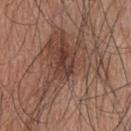Q: Is there a histopathology result?
A: total-body-photography surveillance lesion; no biopsy
Q: What kind of image is this?
A: ~15 mm crop, total-body skin-cancer survey
Q: Automated lesion metrics?
A: a lesion area of about 55 mm² and an eccentricity of roughly 0.85; a mean CIELAB color near L≈45 a*≈18 b*≈25, roughly 8 lightness units darker than nearby skin, and a lesion-to-skin contrast of about 6.5 (normalized; higher = more distinct); a border-irregularity rating of about 9/10 and radial color variation of about 2; a classifier nevus-likeness of about 65/100
Q: What lighting was used for the tile?
A: white-light
Q: Lesion size?
A: ~15 mm (longest diameter)
Q: Who is the patient?
A: male, in their mid- to late 40s
Q: Lesion location?
A: the back